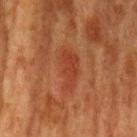– size · ≈5.5 mm
– image · ~15 mm tile from a whole-body skin photo
– tile lighting · cross-polarized
– subject · female, aged around 50
– location · the arm